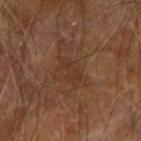| feature | finding |
|---|---|
| biopsy status | catalogued during a skin exam; not biopsied |
| illumination | cross-polarized illumination |
| anatomic site | the right upper arm |
| patient | male, aged around 70 |
| size | ≈3.5 mm |
| image source | ~15 mm tile from a whole-body skin photo |
| image-analysis metrics | a footprint of about 5.5 mm², a shape eccentricity near 0.85, and a symmetry-axis asymmetry near 0.3; a mean CIELAB color near L≈33 a*≈19 b*≈27 and about 5 CIELAB-L* units darker than the surrounding skin; a border-irregularity rating of about 3.5/10, internal color variation of about 1.5 on a 0–10 scale, and radial color variation of about 0.5; an automated nevus-likeness rating near 0 out of 100 and lesion-presence confidence of about 95/100 |